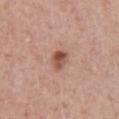This lesion was catalogued during total-body skin photography and was not selected for biopsy. Automated image analysis of the tile measured a lesion area of about 4.5 mm², an outline eccentricity of about 0.6 (0 = round, 1 = elongated), and a shape-asymmetry score of about 0.2 (0 = symmetric). And it measured a lesion color around L≈52 a*≈23 b*≈29 in CIELAB, a lesion–skin lightness drop of about 12, and a lesion-to-skin contrast of about 9 (normalized; higher = more distinct). It also reported a classifier nevus-likeness of about 90/100 and a lesion-detection confidence of about 100/100. A close-up tile cropped from a whole-body skin photograph, about 15 mm across. The subject is a male aged 58 to 62. Captured under white-light illumination. From the chest.